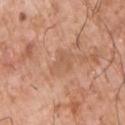The lesion was photographed on a routine skin check and not biopsied; there is no pathology result. This is a white-light tile. A male patient, aged approximately 75. A 15 mm close-up tile from a total-body photography series done for melanoma screening. The lesion-visualizer software estimated an eccentricity of roughly 0.65 and two-axis asymmetry of about 0.4. It also reported an average lesion color of about L≈58 a*≈22 b*≈33 (CIELAB), about 7 CIELAB-L* units darker than the surrounding skin, and a lesion-to-skin contrast of about 4.5 (normalized; higher = more distinct). The software also gave a color-variation rating of about 1.5/10 and a peripheral color-asymmetry measure near 0.5. And it measured a classifier nevus-likeness of about 0/100 and lesion-presence confidence of about 100/100. Longest diameter approximately 3 mm. The lesion is located on the front of the torso.